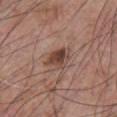Impression: Imaged during a routine full-body skin examination; the lesion was not biopsied and no histopathology is available. Background: A lesion tile, about 15 mm wide, cut from a 3D total-body photograph. On the front of the torso. A male patient aged around 70.